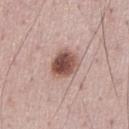Clinical impression:
This lesion was catalogued during total-body skin photography and was not selected for biopsy.
Background:
The total-body-photography lesion software estimated a footprint of about 9 mm², a shape eccentricity near 0.4, and a shape-asymmetry score of about 0.2 (0 = symmetric). The analysis additionally found a border-irregularity index near 2/10 and internal color variation of about 6.5 on a 0–10 scale. From the front of the torso. A male patient, roughly 50 years of age. The tile uses white-light illumination. A 15 mm close-up extracted from a 3D total-body photography capture. Longest diameter approximately 3.5 mm.